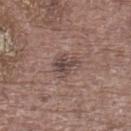Context: Automated tile analysis of the lesion measured an area of roughly 7 mm² and two-axis asymmetry of about 0.3. And it measured a mean CIELAB color near L≈45 a*≈16 b*≈20, about 9 CIELAB-L* units darker than the surrounding skin, and a normalized border contrast of about 7. From the left lower leg. Approximately 3.5 mm at its widest. The tile uses white-light illumination. The patient is a male in their mid- to late 70s. A roughly 15 mm field-of-view crop from a total-body skin photograph.